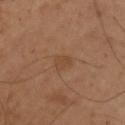{"biopsy_status": "not biopsied; imaged during a skin examination"}